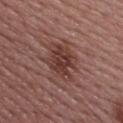notes: catalogued during a skin exam; not biopsied
imaging modality: ~15 mm crop, total-body skin-cancer survey
tile lighting: white-light illumination
location: the upper back
automated lesion analysis: an average lesion color of about L≈39 a*≈21 b*≈23 (CIELAB) and about 9 CIELAB-L* units darker than the surrounding skin; an automated nevus-likeness rating near 50 out of 100 and a lesion-detection confidence of about 100/100
patient: female, approximately 55 years of age